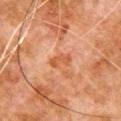Assessment:
This lesion was catalogued during total-body skin photography and was not selected for biopsy.
Image and clinical context:
Imaged with cross-polarized lighting. A close-up tile cropped from a whole-body skin photograph, about 15 mm across. The recorded lesion diameter is about 3 mm. The lesion is on the chest. The subject is a male aged approximately 80.